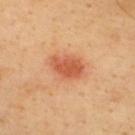Q: Was a biopsy performed?
A: imaged on a skin check; not biopsied
Q: How large is the lesion?
A: about 4 mm
Q: What kind of image is this?
A: ~15 mm tile from a whole-body skin photo
Q: What did automated image analysis measure?
A: a lesion color around L≈58 a*≈30 b*≈38 in CIELAB and a normalized border contrast of about 7.5; a nevus-likeness score of about 100/100
Q: Illumination type?
A: cross-polarized
Q: What are the patient's age and sex?
A: male, aged 38 to 42
Q: Lesion location?
A: the upper back